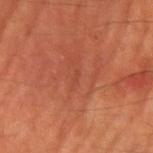biopsy status: catalogued during a skin exam; not biopsied
patient: male, aged 63 to 67
acquisition: ~15 mm tile from a whole-body skin photo
body site: the right upper arm